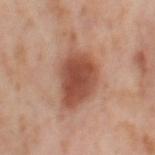No biopsy was performed on this lesion — it was imaged during a full skin examination and was not determined to be concerning. This is a cross-polarized tile. A female patient in their mid- to late 50s. The lesion's longest dimension is about 7.5 mm. Located on the right thigh. A roughly 15 mm field-of-view crop from a total-body skin photograph.